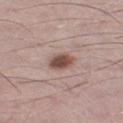This lesion was catalogued during total-body skin photography and was not selected for biopsy.
Approximately 3 mm at its widest.
The tile uses white-light illumination.
On the left thigh.
A region of skin cropped from a whole-body photographic capture, roughly 15 mm wide.
A male patient aged 48 to 52.